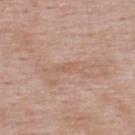{"biopsy_status": "not biopsied; imaged during a skin examination", "lesion_size": {"long_diameter_mm_approx": 3.0}, "patient": {"sex": "male", "age_approx": 55}, "lighting": "white-light", "site": "upper back", "image": {"source": "total-body photography crop", "field_of_view_mm": 15}}